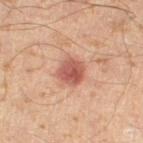follow-up=imaged on a skin check; not biopsied | location=the leg | automated lesion analysis=border irregularity of about 1.5 on a 0–10 scale and a peripheral color-asymmetry measure near 1.5 | image source=15 mm crop, total-body photography | size=~3.5 mm (longest diameter) | subject=male, in their mid-60s.